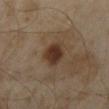No biopsy was performed on this lesion — it was imaged during a full skin examination and was not determined to be concerning. The subject is a male approximately 60 years of age. Automated tile analysis of the lesion measured a lesion area of about 6.5 mm² and a symmetry-axis asymmetry near 0.15. And it measured an average lesion color of about L≈29 a*≈15 b*≈23 (CIELAB), about 11 CIELAB-L* units darker than the surrounding skin, and a normalized border contrast of about 11. And it measured a border-irregularity index near 1.5/10, internal color variation of about 2.5 on a 0–10 scale, and radial color variation of about 1. The analysis additionally found a classifier nevus-likeness of about 95/100 and a lesion-detection confidence of about 100/100. A 15 mm close-up extracted from a 3D total-body photography capture. The recorded lesion diameter is about 3 mm. Located on the abdomen. This is a cross-polarized tile.